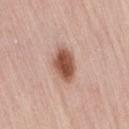Assessment:
The lesion was tiled from a total-body skin photograph and was not biopsied.
Clinical summary:
The patient is a female approximately 65 years of age. Cropped from a total-body skin-imaging series; the visible field is about 15 mm. The lesion-visualizer software estimated a mean CIELAB color near L≈53 a*≈23 b*≈29 and a lesion-to-skin contrast of about 11 (normalized; higher = more distinct). And it measured a border-irregularity index near 1.5/10 and internal color variation of about 4.5 on a 0–10 scale. The analysis additionally found an automated nevus-likeness rating near 100 out of 100. Located on the right thigh.